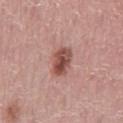image source: ~15 mm crop, total-body skin-cancer survey
location: the mid back
automated lesion analysis: an automated nevus-likeness rating near 85 out of 100
patient: male, about 70 years old